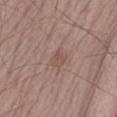Captured during whole-body skin photography for melanoma surveillance; the lesion was not biopsied. A roughly 15 mm field-of-view crop from a total-body skin photograph. The subject is a male aged around 65. The tile uses white-light illumination. The lesion's longest dimension is about 2.5 mm. Located on the right forearm.